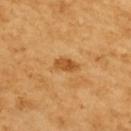<record>
  <biopsy_status>not biopsied; imaged during a skin examination</biopsy_status>
  <site>upper back</site>
  <lesion_size>
    <long_diameter_mm_approx>3.0</long_diameter_mm_approx>
  </lesion_size>
  <patient>
    <sex>female</sex>
    <age_approx>55</age_approx>
  </patient>
  <image>
    <source>total-body photography crop</source>
    <field_of_view_mm>15</field_of_view_mm>
  </image>
  <lighting>cross-polarized</lighting>
</record>